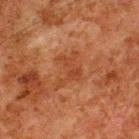Assessment: Recorded during total-body skin imaging; not selected for excision or biopsy. Background: Cropped from a total-body skin-imaging series; the visible field is about 15 mm. The lesion is located on the upper back. This is a cross-polarized tile. The patient is a male in their 80s. About 4.5 mm across.